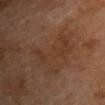workup: catalogued during a skin exam; not biopsied | subject: male, approximately 75 years of age | site: the chest | lesion diameter: about 7 mm | image-analysis metrics: a symmetry-axis asymmetry near 0.5; roughly 4 lightness units darker than nearby skin and a normalized lesion–skin contrast near 5; border irregularity of about 9 on a 0–10 scale and a within-lesion color-variation index near 2.5/10; a nevus-likeness score of about 0/100 and a detector confidence of about 100 out of 100 that the crop contains a lesion | image source: ~15 mm tile from a whole-body skin photo.